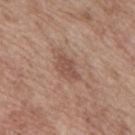workup: imaged on a skin check; not biopsied
body site: the mid back
lighting: white-light illumination
lesion diameter: ~3.5 mm (longest diameter)
subject: male, about 70 years old
TBP lesion metrics: an average lesion color of about L≈50 a*≈21 b*≈26 (CIELAB), roughly 9 lightness units darker than nearby skin, and a normalized border contrast of about 6.5; a border-irregularity index near 3/10, a within-lesion color-variation index near 1.5/10, and a peripheral color-asymmetry measure near 0.5; a classifier nevus-likeness of about 40/100
image source: ~15 mm tile from a whole-body skin photo